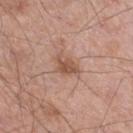Captured during whole-body skin photography for melanoma surveillance; the lesion was not biopsied.
The subject is a male aged around 55.
The lesion is on the right thigh.
A 15 mm close-up extracted from a 3D total-body photography capture.